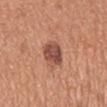Case summary:
• biopsy status: no biopsy performed (imaged during a skin exam)
• illumination: white-light illumination
• patient: male, in their mid- to late 60s
• lesion diameter: ≈4 mm
• image: total-body-photography crop, ~15 mm field of view
• location: the mid back